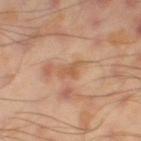Context: The lesion-visualizer software estimated a lesion–skin lightness drop of about 8 and a normalized lesion–skin contrast near 6. And it measured a border-irregularity index near 4.5/10, a color-variation rating of about 1/10, and radial color variation of about 0.5. It also reported an automated nevus-likeness rating near 0 out of 100 and a lesion-detection confidence of about 100/100. A 15 mm close-up extracted from a 3D total-body photography capture. Approximately 2.5 mm at its widest. From the right thigh. A male patient, approximately 45 years of age.